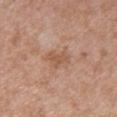Clinical impression:
Imaged during a routine full-body skin examination; the lesion was not biopsied and no histopathology is available.
Clinical summary:
This is a white-light tile. A 15 mm close-up tile from a total-body photography series done for melanoma screening. From the upper back. The recorded lesion diameter is about 3 mm. The total-body-photography lesion software estimated a footprint of about 4.5 mm² and a shape-asymmetry score of about 0.4 (0 = symmetric). And it measured a lesion color around L≈55 a*≈21 b*≈32 in CIELAB and a normalized border contrast of about 6.5. The analysis additionally found a border-irregularity index near 4.5/10, a within-lesion color-variation index near 1/10, and a peripheral color-asymmetry measure near 0.5. It also reported a classifier nevus-likeness of about 0/100 and lesion-presence confidence of about 100/100. A female patient aged around 30.